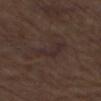Clinical impression: The lesion was photographed on a routine skin check and not biopsied; there is no pathology result. Acquisition and patient details: The lesion-visualizer software estimated an area of roughly 7.5 mm², an outline eccentricity of about 0.95 (0 = round, 1 = elongated), and two-axis asymmetry of about 0.5. The software also gave an average lesion color of about L≈27 a*≈13 b*≈16 (CIELAB), a lesion–skin lightness drop of about 4, and a normalized lesion–skin contrast near 6. It also reported a nevus-likeness score of about 0/100 and a detector confidence of about 55 out of 100 that the crop contains a lesion. Imaged with white-light lighting. Cropped from a whole-body photographic skin survey; the tile spans about 15 mm. About 5.5 mm across. From the left thigh. The patient is a male aged 73–77.